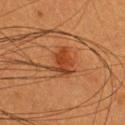Part of a total-body skin-imaging series; this lesion was reviewed on a skin check and was not flagged for biopsy. Automated image analysis of the tile measured a lesion color around L≈37 a*≈25 b*≈34 in CIELAB, roughly 8 lightness units darker than nearby skin, and a normalized lesion–skin contrast near 7.5. It also reported a border-irregularity rating of about 3.5/10 and a within-lesion color-variation index near 4/10. Captured under cross-polarized illumination. A female patient, about 55 years old. A close-up tile cropped from a whole-body skin photograph, about 15 mm across. Located on the upper back. The lesion's longest dimension is about 3.5 mm.